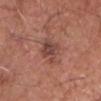Recorded during total-body skin imaging; not selected for excision or biopsy. Imaged with white-light lighting. The lesion is on the head or neck. Approximately 3.5 mm at its widest. A lesion tile, about 15 mm wide, cut from a 3D total-body photograph. A male subject, about 80 years old.